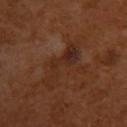| feature | finding |
|---|---|
| notes | catalogued during a skin exam; not biopsied |
| patient | female, aged approximately 55 |
| image | ~15 mm tile from a whole-body skin photo |
| size | ≈6 mm |
| image-analysis metrics | a lesion area of about 9 mm², a shape eccentricity near 0.95, and a shape-asymmetry score of about 0.65 (0 = symmetric); a normalized lesion–skin contrast near 6.5; border irregularity of about 10 on a 0–10 scale and a color-variation rating of about 4/10; an automated nevus-likeness rating near 0 out of 100 |
| location | the upper back |